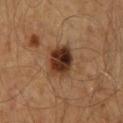Clinical impression:
Recorded during total-body skin imaging; not selected for excision or biopsy.